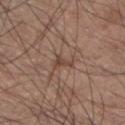notes: total-body-photography surveillance lesion; no biopsy
image-analysis metrics: a lesion color around L≈44 a*≈17 b*≈25 in CIELAB, about 8 CIELAB-L* units darker than the surrounding skin, and a lesion-to-skin contrast of about 6.5 (normalized; higher = more distinct); border irregularity of about 6.5 on a 0–10 scale, a color-variation rating of about 0/10, and radial color variation of about 0
image source: ~15 mm tile from a whole-body skin photo
anatomic site: the left lower leg
lesion diameter: ≈2.5 mm
illumination: white-light illumination
subject: male, in their mid- to late 30s